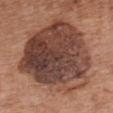notes: catalogued during a skin exam; not biopsied
patient: female, in their mid- to late 60s
acquisition: ~15 mm tile from a whole-body skin photo
lesion diameter: ~9.5 mm (longest diameter)
anatomic site: the front of the torso
illumination: white-light illumination
image-analysis metrics: a shape eccentricity near 0.3 and a shape-asymmetry score of about 0.25 (0 = symmetric)